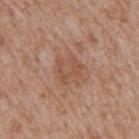Recorded during total-body skin imaging; not selected for excision or biopsy. From the mid back. A male subject approximately 65 years of age. A roughly 15 mm field-of-view crop from a total-body skin photograph.